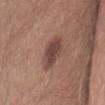Q: Was a biopsy performed?
A: imaged on a skin check; not biopsied
Q: Lesion size?
A: ≈4.5 mm
Q: What are the patient's age and sex?
A: male, in their mid-30s
Q: Illumination type?
A: white-light illumination
Q: Lesion location?
A: the right thigh
Q: What is the imaging modality?
A: total-body-photography crop, ~15 mm field of view
Q: Automated lesion metrics?
A: a within-lesion color-variation index near 2.5/10 and a peripheral color-asymmetry measure near 0.5; an automated nevus-likeness rating near 70 out of 100 and lesion-presence confidence of about 100/100